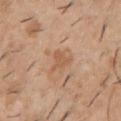Findings:
– biopsy status: imaged on a skin check; not biopsied
– image source: ~15 mm crop, total-body skin-cancer survey
– tile lighting: white-light illumination
– anatomic site: the chest
– patient: male, roughly 40 years of age
– lesion size: ~3 mm (longest diameter)